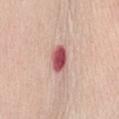Assessment:
The lesion was tiled from a total-body skin photograph and was not biopsied.
Image and clinical context:
The lesion is on the abdomen. About 3.5 mm across. This image is a 15 mm lesion crop taken from a total-body photograph. A female patient in their mid-60s.